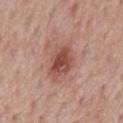Q: Was this lesion biopsied?
A: total-body-photography surveillance lesion; no biopsy
Q: How was the tile lit?
A: white-light illumination
Q: How was this image acquired?
A: total-body-photography crop, ~15 mm field of view
Q: What are the patient's age and sex?
A: male, aged approximately 55
Q: Lesion location?
A: the mid back
Q: How large is the lesion?
A: ≈4.5 mm